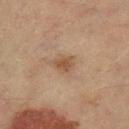No biopsy was performed on this lesion — it was imaged during a full skin examination and was not determined to be concerning.
On the leg.
A female subject, in their mid-50s.
The lesion's longest dimension is about 3 mm.
Cropped from a whole-body photographic skin survey; the tile spans about 15 mm.